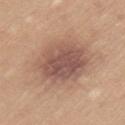workup — total-body-photography surveillance lesion; no biopsy
lesion size — ≈7.5 mm
acquisition — total-body-photography crop, ~15 mm field of view
site — the arm
illumination — white-light
subject — male, about 35 years old